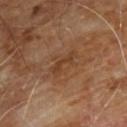Q: Was this lesion biopsied?
A: catalogued during a skin exam; not biopsied
Q: What lighting was used for the tile?
A: cross-polarized
Q: Lesion location?
A: the chest
Q: Lesion size?
A: ~3.5 mm (longest diameter)
Q: Patient demographics?
A: male, roughly 60 years of age
Q: What did automated image analysis measure?
A: a classifier nevus-likeness of about 0/100 and lesion-presence confidence of about 95/100
Q: What is the imaging modality?
A: 15 mm crop, total-body photography